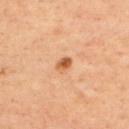From the upper back. A male subject, in their mid-50s. A lesion tile, about 15 mm wide, cut from a 3D total-body photograph. The lesion-visualizer software estimated a border-irregularity rating of about 1.5/10 and internal color variation of about 4.5 on a 0–10 scale. It also reported a classifier nevus-likeness of about 85/100. About 2 mm across. Imaged with cross-polarized lighting.